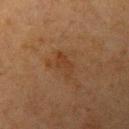Clinical impression:
The lesion was tiled from a total-body skin photograph and was not biopsied.
Context:
The lesion-visualizer software estimated an outline eccentricity of about 0.9 (0 = round, 1 = elongated) and a symmetry-axis asymmetry near 0.4. And it measured an automated nevus-likeness rating near 0 out of 100 and a lesion-detection confidence of about 100/100. On the left upper arm. This is a cross-polarized tile. A female patient aged around 55. A region of skin cropped from a whole-body photographic capture, roughly 15 mm wide. The lesion's longest dimension is about 3 mm.